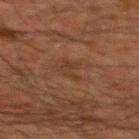Captured during whole-body skin photography for melanoma surveillance; the lesion was not biopsied.
This image is a 15 mm lesion crop taken from a total-body photograph.
Automated image analysis of the tile measured an average lesion color of about L≈28 a*≈16 b*≈24 (CIELAB) and roughly 4 lightness units darker than nearby skin. The software also gave a nevus-likeness score of about 0/100 and lesion-presence confidence of about 100/100.
The patient is a male aged 58–62.
On the back.
Measured at roughly 3 mm in maximum diameter.